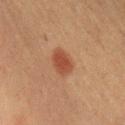Findings:
- follow-up: total-body-photography surveillance lesion; no biopsy
- size: ≈3.5 mm
- lighting: cross-polarized
- anatomic site: the left upper arm
- patient: female, roughly 50 years of age
- acquisition: 15 mm crop, total-body photography
- automated lesion analysis: an area of roughly 7 mm², a shape eccentricity near 0.6, and a shape-asymmetry score of about 0.15 (0 = symmetric); a mean CIELAB color near L≈39 a*≈22 b*≈28, roughly 9 lightness units darker than nearby skin, and a normalized border contrast of about 7.5; a classifier nevus-likeness of about 95/100 and lesion-presence confidence of about 100/100